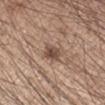location: the right forearm | lesion size: ≈2.5 mm | automated lesion analysis: a footprint of about 4.5 mm², an outline eccentricity of about 0.65 (0 = round, 1 = elongated), and a symmetry-axis asymmetry near 0.3; a border-irregularity index near 2.5/10, a color-variation rating of about 4/10, and a peripheral color-asymmetry measure near 1 | imaging modality: total-body-photography crop, ~15 mm field of view | lighting: white-light | patient: male, aged 33–37.